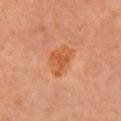Case summary:
- biopsy status · catalogued during a skin exam; not biopsied
- anatomic site · the left arm
- size · ≈4 mm
- imaging modality · ~15 mm crop, total-body skin-cancer survey
- patient · female, in their mid- to late 60s
- tile lighting · cross-polarized